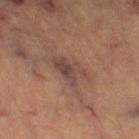The lesion was tiled from a total-body skin photograph and was not biopsied.
The lesion is on the leg.
A female patient, approximately 65 years of age.
Approximately 4 mm at its widest.
Cropped from a whole-body photographic skin survey; the tile spans about 15 mm.